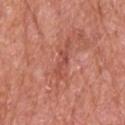Q: Is there a histopathology result?
A: catalogued during a skin exam; not biopsied
Q: Lesion location?
A: the head or neck
Q: What lighting was used for the tile?
A: white-light illumination
Q: Automated lesion metrics?
A: a footprint of about 2.5 mm², an outline eccentricity of about 0.95 (0 = round, 1 = elongated), and a symmetry-axis asymmetry near 0.45; border irregularity of about 6.5 on a 0–10 scale and a color-variation rating of about 0/10
Q: What is the imaging modality?
A: ~15 mm tile from a whole-body skin photo
Q: What is the lesion's diameter?
A: ~3 mm (longest diameter)
Q: What are the patient's age and sex?
A: male, roughly 80 years of age